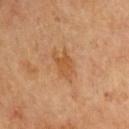The lesion was tiled from a total-body skin photograph and was not biopsied. Cropped from a whole-body photographic skin survey; the tile spans about 15 mm. Longest diameter approximately 3.5 mm. On the arm. The patient is a male roughly 65 years of age.